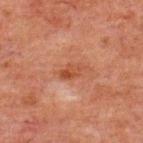Captured under cross-polarized illumination.
Approximately 3 mm at its widest.
Located on the upper back.
A male patient approximately 60 years of age.
A 15 mm close-up tile from a total-body photography series done for melanoma screening.